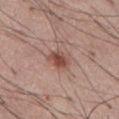Notes:
* workup — catalogued during a skin exam; not biopsied
* subject — male, aged 53–57
* size — ~3 mm (longest diameter)
* image — ~15 mm tile from a whole-body skin photo
* body site — the abdomen
* image-analysis metrics — a lesion color around L≈49 a*≈22 b*≈26 in CIELAB, a lesion–skin lightness drop of about 11, and a normalized border contrast of about 8.5
* illumination — white-light